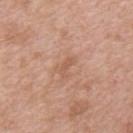Q: Was a biopsy performed?
A: catalogued during a skin exam; not biopsied
Q: What is the lesion's diameter?
A: about 2.5 mm
Q: What is the imaging modality?
A: ~15 mm tile from a whole-body skin photo
Q: Where on the body is the lesion?
A: the mid back
Q: Illumination type?
A: white-light
Q: Who is the patient?
A: male, approximately 50 years of age